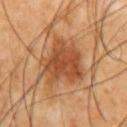Notes:
- follow-up · catalogued during a skin exam; not biopsied
- site · the chest
- diameter · about 6 mm
- subject · male, roughly 65 years of age
- tile lighting · cross-polarized
- imaging modality · 15 mm crop, total-body photography